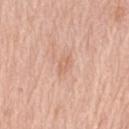Clinical impression: This lesion was catalogued during total-body skin photography and was not selected for biopsy. Background: A region of skin cropped from a whole-body photographic capture, roughly 15 mm wide. Imaged with white-light lighting. The lesion is located on the right upper arm. The patient is a female in their 70s.